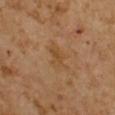A lesion tile, about 15 mm wide, cut from a 3D total-body photograph. A male subject, aged 63–67.